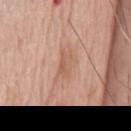  biopsy_status: not biopsied; imaged during a skin examination
  automated_metrics:
    area_mm2_approx: 4.0
    eccentricity: 0.9
    border_irregularity_0_10: 4.0
    peripheral_color_asymmetry: 0.5
  lesion_size:
    long_diameter_mm_approx: 3.5
  image:
    source: total-body photography crop
    field_of_view_mm: 15
  lighting: white-light
  site: chest
  patient:
    sex: male
    age_approx: 75